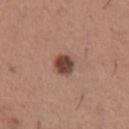<tbp_lesion>
  <biopsy_status>not biopsied; imaged during a skin examination</biopsy_status>
  <patient>
    <sex>male</sex>
    <age_approx>45</age_approx>
  </patient>
  <lighting>white-light</lighting>
  <site>front of the torso</site>
  <lesion_size>
    <long_diameter_mm_approx>2.5</long_diameter_mm_approx>
  </lesion_size>
  <image>
    <source>total-body photography crop</source>
    <field_of_view_mm>15</field_of_view_mm>
  </image>
</tbp_lesion>